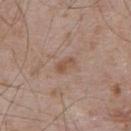follow-up: no biopsy performed (imaged during a skin exam)
subject: male, in their mid-50s
location: the upper back
tile lighting: white-light illumination
image source: ~15 mm tile from a whole-body skin photo